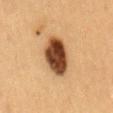patient: female, approximately 40 years of age
image: ~15 mm tile from a whole-body skin photo
location: the mid back